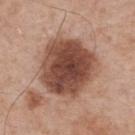Recorded during total-body skin imaging; not selected for excision or biopsy. Imaged with white-light lighting. A region of skin cropped from a whole-body photographic capture, roughly 15 mm wide. The total-body-photography lesion software estimated a footprint of about 35 mm², a shape eccentricity near 0.4, and two-axis asymmetry of about 0.2. The software also gave an average lesion color of about L≈47 a*≈21 b*≈27 (CIELAB) and a normalized border contrast of about 12. A male subject in their mid-50s. Located on the upper back.